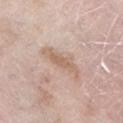The lesion was photographed on a routine skin check and not biopsied; there is no pathology result. The lesion is located on the left lower leg. A female subject aged 68 to 72. Imaged with white-light lighting. An algorithmic analysis of the crop reported an area of roughly 7.5 mm², an eccentricity of roughly 0.95, and a shape-asymmetry score of about 0.35 (0 = symmetric). The analysis additionally found about 9 CIELAB-L* units darker than the surrounding skin. The analysis additionally found an automated nevus-likeness rating near 10 out of 100 and lesion-presence confidence of about 75/100. Cropped from a total-body skin-imaging series; the visible field is about 15 mm.